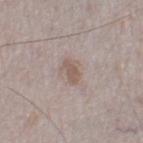workup=no biopsy performed (imaged during a skin exam); site=the right thigh; imaging modality=total-body-photography crop, ~15 mm field of view; lesion diameter=~3 mm (longest diameter); lighting=white-light; subject=male, approximately 75 years of age.